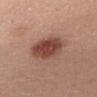Case summary:
• biopsy status: imaged on a skin check; not biopsied
• subject: female, aged around 35
• image-analysis metrics: a color-variation rating of about 5/10 and a peripheral color-asymmetry measure near 1.5; a nevus-likeness score of about 100/100 and lesion-presence confidence of about 100/100
• body site: the chest
• lesion diameter: about 5 mm
• illumination: white-light illumination
• image source: ~15 mm tile from a whole-body skin photo